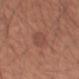Q: Was this lesion biopsied?
A: imaged on a skin check; not biopsied
Q: What are the patient's age and sex?
A: male, in their mid- to late 40s
Q: What kind of image is this?
A: 15 mm crop, total-body photography
Q: What is the anatomic site?
A: the left forearm
Q: How large is the lesion?
A: about 4 mm